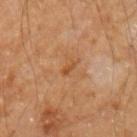Q: What kind of image is this?
A: ~15 mm crop, total-body skin-cancer survey
Q: How large is the lesion?
A: about 2 mm
Q: What lighting was used for the tile?
A: cross-polarized
Q: Lesion location?
A: the left upper arm
Q: What did automated image analysis measure?
A: a lesion area of about 2 mm², an outline eccentricity of about 0.9 (0 = round, 1 = elongated), and a symmetry-axis asymmetry near 0.25; a classifier nevus-likeness of about 0/100 and a detector confidence of about 100 out of 100 that the crop contains a lesion
Q: Who is the patient?
A: male, roughly 60 years of age